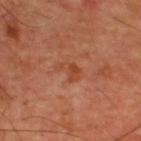notes: total-body-photography surveillance lesion; no biopsy
subject: male, aged 58–62
location: the upper back
imaging modality: 15 mm crop, total-body photography
tile lighting: cross-polarized
size: ≈3 mm